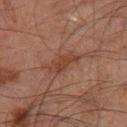follow-up = total-body-photography surveillance lesion; no biopsy | body site = the right thigh | diameter = about 4 mm | patient = male, approximately 70 years of age | illumination = cross-polarized | acquisition = ~15 mm tile from a whole-body skin photo | TBP lesion metrics = a mean CIELAB color near L≈33 a*≈18 b*≈25, a lesion–skin lightness drop of about 6, and a normalized border contrast of about 6.5; a border-irregularity index near 4/10, a within-lesion color-variation index near 1.5/10, and radial color variation of about 0.5.